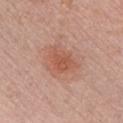biopsy status — no biopsy performed (imaged during a skin exam) | site — the chest | diameter — about 4 mm | image-analysis metrics — a shape eccentricity near 0.55 and two-axis asymmetry of about 0.4; roughly 8 lightness units darker than nearby skin and a lesion-to-skin contrast of about 6 (normalized; higher = more distinct); radial color variation of about 1 | patient — male, in their 30s | image — 15 mm crop, total-body photography | illumination — white-light illumination.